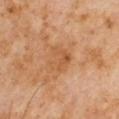Case summary:
- notes · catalogued during a skin exam; not biopsied
- size · ≈3 mm
- subject · male, in their 60s
- illumination · cross-polarized
- anatomic site · the arm
- image source · total-body-photography crop, ~15 mm field of view